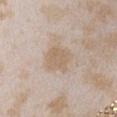Captured during whole-body skin photography for melanoma surveillance; the lesion was not biopsied. This image is a 15 mm lesion crop taken from a total-body photograph. The total-body-photography lesion software estimated border irregularity of about 2.5 on a 0–10 scale, internal color variation of about 2.5 on a 0–10 scale, and peripheral color asymmetry of about 1. And it measured an automated nevus-likeness rating near 20 out of 100 and a detector confidence of about 100 out of 100 that the crop contains a lesion. A female subject aged approximately 25. The tile uses white-light illumination. The lesion's longest dimension is about 3.5 mm. From the chest.